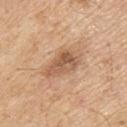  biopsy_status: not biopsied; imaged during a skin examination
  site: upper back
  patient:
    sex: male
    age_approx: 70
  image:
    source: total-body photography crop
    field_of_view_mm: 15
  lighting: white-light
  lesion_size:
    long_diameter_mm_approx: 4.5